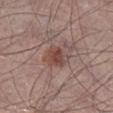| feature | finding |
|---|---|
| biopsy status | total-body-photography surveillance lesion; no biopsy |
| image-analysis metrics | a lesion area of about 7 mm² and a symmetry-axis asymmetry near 0.3; about 9 CIELAB-L* units darker than the surrounding skin; internal color variation of about 4 on a 0–10 scale; an automated nevus-likeness rating near 80 out of 100 and a detector confidence of about 100 out of 100 that the crop contains a lesion |
| diameter | ≈3.5 mm |
| site | the right lower leg |
| imaging modality | total-body-photography crop, ~15 mm field of view |
| illumination | white-light illumination |
| subject | male, aged approximately 65 |